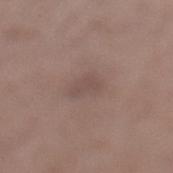Assessment: This lesion was catalogued during total-body skin photography and was not selected for biopsy. Image and clinical context: From the left lower leg. A male patient aged 48–52. A roughly 15 mm field-of-view crop from a total-body skin photograph. The tile uses white-light illumination.